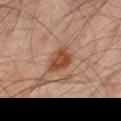image: ~15 mm tile from a whole-body skin photo | site: the right thigh | image-analysis metrics: a mean CIELAB color near L≈36 a*≈18 b*≈26, roughly 8 lightness units darker than nearby skin, and a lesion-to-skin contrast of about 9 (normalized; higher = more distinct); a nevus-likeness score of about 85/100 | patient: male, approximately 50 years of age | lesion size: ~4 mm (longest diameter).